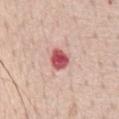| key | value |
|---|---|
| biopsy status | imaged on a skin check; not biopsied |
| acquisition | ~15 mm tile from a whole-body skin photo |
| site | the chest |
| subject | male, in their 60s |